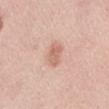Findings:
– tile lighting · white-light illumination
– subject · female, in their 50s
– acquisition · ~15 mm tile from a whole-body skin photo
– lesion size · ≈3 mm
– anatomic site · the leg